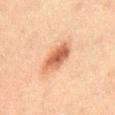Context: The tile uses cross-polarized illumination. A male subject, in their 50s. Located on the abdomen. A 15 mm crop from a total-body photograph taken for skin-cancer surveillance.